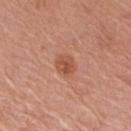workup: no biopsy performed (imaged during a skin exam)
diameter: ≈2.5 mm
patient: female, aged around 75
illumination: white-light
site: the right upper arm
TBP lesion metrics: a lesion area of about 4.5 mm² and an eccentricity of roughly 0.5; a within-lesion color-variation index near 3/10 and peripheral color asymmetry of about 1
imaging modality: 15 mm crop, total-body photography